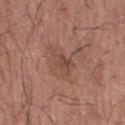Notes:
• follow-up — catalogued during a skin exam; not biopsied
• anatomic site — the right forearm
• imaging modality — 15 mm crop, total-body photography
• subject — male, in their mid- to late 70s